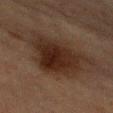biopsy status: imaged on a skin check; not biopsied
tile lighting: cross-polarized illumination
patient: male, approximately 75 years of age
automated lesion analysis: a border-irregularity index near 3.5/10 and a within-lesion color-variation index near 4/10
diameter: about 7 mm
anatomic site: the arm
image: ~15 mm crop, total-body skin-cancer survey